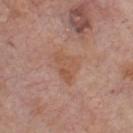No biopsy was performed on this lesion — it was imaged during a full skin examination and was not determined to be concerning.
A male subject, aged 73 to 77.
The lesion is located on the chest.
A 15 mm close-up tile from a total-body photography series done for melanoma screening.
The lesion-visualizer software estimated an eccentricity of roughly 0.75. The software also gave a mean CIELAB color near L≈53 a*≈21 b*≈30, about 6 CIELAB-L* units darker than the surrounding skin, and a lesion-to-skin contrast of about 5.5 (normalized; higher = more distinct). The analysis additionally found a detector confidence of about 100 out of 100 that the crop contains a lesion.